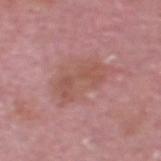Captured during whole-body skin photography for melanoma surveillance; the lesion was not biopsied.
A 15 mm crop from a total-body photograph taken for skin-cancer surveillance.
The tile uses white-light illumination.
The total-body-photography lesion software estimated a lesion area of about 8 mm², an outline eccentricity of about 0.9 (0 = round, 1 = elongated), and a symmetry-axis asymmetry near 0.5. And it measured a border-irregularity rating of about 8/10, a color-variation rating of about 2.5/10, and radial color variation of about 1. The software also gave a nevus-likeness score of about 0/100 and a lesion-detection confidence of about 100/100.
About 5 mm across.
The patient is a male aged 63–67.
On the head or neck.